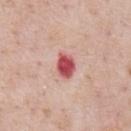Q: Was a biopsy performed?
A: no biopsy performed (imaged during a skin exam)
Q: What is the lesion's diameter?
A: ~3 mm (longest diameter)
Q: What are the patient's age and sex?
A: male, roughly 60 years of age
Q: What is the anatomic site?
A: the abdomen
Q: How was the tile lit?
A: white-light illumination
Q: How was this image acquired?
A: 15 mm crop, total-body photography
Q: Automated lesion metrics?
A: a lesion color around L≈54 a*≈35 b*≈24 in CIELAB and roughly 17 lightness units darker than nearby skin